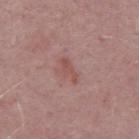<lesion>
<biopsy_status>not biopsied; imaged during a skin examination</biopsy_status>
<site>back</site>
<image>
  <source>total-body photography crop</source>
  <field_of_view_mm>15</field_of_view_mm>
</image>
<patient>
  <sex>male</sex>
  <age_approx>50</age_approx>
</patient>
<lighting>white-light</lighting>
<lesion_size>
  <long_diameter_mm_approx>3.0</long_diameter_mm_approx>
</lesion_size>
</lesion>